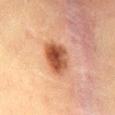Clinical impression:
Imaged during a routine full-body skin examination; the lesion was not biopsied and no histopathology is available.
Acquisition and patient details:
A 15 mm crop from a total-body photograph taken for skin-cancer surveillance. The subject is a male aged approximately 60. The lesion is located on the mid back.